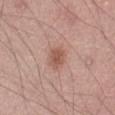No biopsy was performed on this lesion — it was imaged during a full skin examination and was not determined to be concerning. Automated image analysis of the tile measured a color-variation rating of about 2.5/10 and radial color variation of about 1. On the front of the torso. A male subject roughly 75 years of age. A lesion tile, about 15 mm wide, cut from a 3D total-body photograph. Approximately 3 mm at its widest.